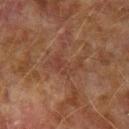Notes:
- notes: no biopsy performed (imaged during a skin exam)
- location: the arm
- patient: male, in their mid- to late 70s
- TBP lesion metrics: two-axis asymmetry of about 0.5; a lesion color around L≈33 a*≈18 b*≈24 in CIELAB, roughly 5 lightness units darker than nearby skin, and a normalized lesion–skin contrast near 5; a detector confidence of about 55 out of 100 that the crop contains a lesion
- image: ~15 mm tile from a whole-body skin photo